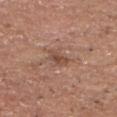Q: How was this image acquired?
A: ~15 mm tile from a whole-body skin photo
Q: Who is the patient?
A: male, roughly 70 years of age
Q: What is the anatomic site?
A: the head or neck
Q: Lesion size?
A: ~2.5 mm (longest diameter)